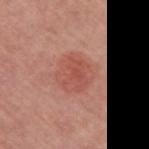Automated image analysis of the tile measured an area of roughly 13 mm², an outline eccentricity of about 0.4 (0 = round, 1 = elongated), and a shape-asymmetry score of about 0.15 (0 = symmetric). And it measured a lesion–skin lightness drop of about 7 and a lesion-to-skin contrast of about 5.5 (normalized; higher = more distinct). It also reported border irregularity of about 2 on a 0–10 scale. And it measured a nevus-likeness score of about 50/100. Located on the right upper arm. A female patient, in their mid-60s. Imaged with white-light lighting. A lesion tile, about 15 mm wide, cut from a 3D total-body photograph. Longest diameter approximately 4 mm.